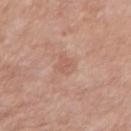Imaged with white-light lighting.
About 3 mm across.
This image is a 15 mm lesion crop taken from a total-body photograph.
A male subject, roughly 55 years of age.
The lesion is located on the right upper arm.